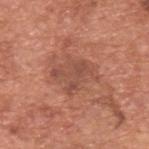| field | value |
|---|---|
| follow-up | imaged on a skin check; not biopsied |
| automated metrics | a footprint of about 12 mm², an eccentricity of roughly 0.65, and a shape-asymmetry score of about 0.4 (0 = symmetric); internal color variation of about 3 on a 0–10 scale and peripheral color asymmetry of about 1 |
| acquisition | 15 mm crop, total-body photography |
| patient | male, aged approximately 65 |
| lesion diameter | ~4.5 mm (longest diameter) |
| location | the back |